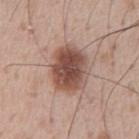{
  "biopsy_status": "not biopsied; imaged during a skin examination",
  "patient": {
    "sex": "male",
    "age_approx": 55
  },
  "lesion_size": {
    "long_diameter_mm_approx": 5.5
  },
  "image": {
    "source": "total-body photography crop",
    "field_of_view_mm": 15
  },
  "automated_metrics": {
    "area_mm2_approx": 18.0,
    "border_irregularity_0_10": 1.5,
    "color_variation_0_10": 6.0,
    "nevus_likeness_0_100": 80,
    "lesion_detection_confidence_0_100": 100
  },
  "site": "abdomen",
  "lighting": "white-light"
}